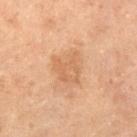Findings:
– notes — no biopsy performed (imaged during a skin exam)
– location — the abdomen
– size — ≈3.5 mm
– tile lighting — cross-polarized illumination
– image — 15 mm crop, total-body photography
– automated metrics — an automated nevus-likeness rating near 0 out of 100
– patient — male, aged around 70